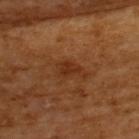follow-up: total-body-photography surveillance lesion; no biopsy | TBP lesion metrics: a classifier nevus-likeness of about 0/100 and lesion-presence confidence of about 100/100 | image: 15 mm crop, total-body photography | lesion diameter: about 3.5 mm | anatomic site: the upper back | patient: male, in their mid-60s.